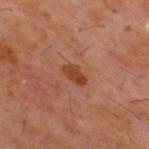• image: ~15 mm crop, total-body skin-cancer survey
• tile lighting: cross-polarized
• lesion diameter: about 3 mm
• body site: the upper back
• patient: male, in their 60s